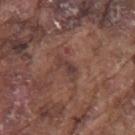Q: Was a biopsy performed?
A: total-body-photography surveillance lesion; no biopsy
Q: What is the imaging modality?
A: total-body-photography crop, ~15 mm field of view
Q: What is the anatomic site?
A: the left upper arm
Q: What are the patient's age and sex?
A: male, roughly 75 years of age